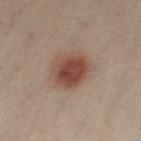Part of a total-body skin-imaging series; this lesion was reviewed on a skin check and was not flagged for biopsy.
A female patient, in their 40s.
A lesion tile, about 15 mm wide, cut from a 3D total-body photograph.
This is a cross-polarized tile.
The lesion is on the right leg.
About 4.5 mm across.